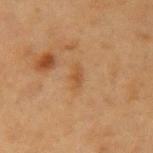Recorded during total-body skin imaging; not selected for excision or biopsy. Cropped from a total-body skin-imaging series; the visible field is about 15 mm. The total-body-photography lesion software estimated a footprint of about 2.5 mm², an eccentricity of roughly 0.9, and two-axis asymmetry of about 0.3. It also reported a nevus-likeness score of about 0/100 and a detector confidence of about 100 out of 100 that the crop contains a lesion. On the left upper arm. Measured at roughly 2.5 mm in maximum diameter. Imaged with cross-polarized lighting. A female patient approximately 40 years of age.